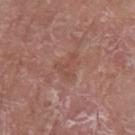Captured during whole-body skin photography for melanoma surveillance; the lesion was not biopsied. On the left lower leg. The patient is a male in their mid- to late 60s. The tile uses white-light illumination. The recorded lesion diameter is about 3 mm. A 15 mm close-up extracted from a 3D total-body photography capture.